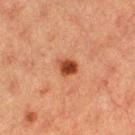Impression: Captured during whole-body skin photography for melanoma surveillance; the lesion was not biopsied. Acquisition and patient details: A 15 mm crop from a total-body photograph taken for skin-cancer surveillance. This is a cross-polarized tile. A female subject aged 38 to 42. Automated image analysis of the tile measured a lesion area of about 4 mm² and an eccentricity of roughly 0.6. The lesion is on the right thigh. Approximately 2.5 mm at its widest.